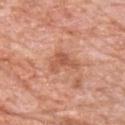biopsy status=no biopsy performed (imaged during a skin exam)
lesion diameter=~3.5 mm (longest diameter)
acquisition=total-body-photography crop, ~15 mm field of view
tile lighting=white-light
patient=male, aged 78 to 82
TBP lesion metrics=a footprint of about 5 mm² and two-axis asymmetry of about 0.6; an average lesion color of about L≈56 a*≈26 b*≈33 (CIELAB) and a normalized lesion–skin contrast near 6.5; an automated nevus-likeness rating near 10 out of 100 and a detector confidence of about 100 out of 100 that the crop contains a lesion
body site=the front of the torso